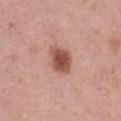{
  "biopsy_status": "not biopsied; imaged during a skin examination",
  "image": {
    "source": "total-body photography crop",
    "field_of_view_mm": 15
  },
  "patient": {
    "sex": "female",
    "age_approx": 40
  },
  "lighting": "white-light",
  "site": "left thigh",
  "automated_metrics": {
    "area_mm2_approx": 8.0,
    "eccentricity": 0.5,
    "shape_asymmetry": 0.2,
    "border_irregularity_0_10": 1.5,
    "color_variation_0_10": 3.5,
    "peripheral_color_asymmetry": 1.0
  },
  "lesion_size": {
    "long_diameter_mm_approx": 3.0
  }
}